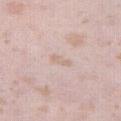Part of a total-body skin-imaging series; this lesion was reviewed on a skin check and was not flagged for biopsy. This image is a 15 mm lesion crop taken from a total-body photograph. The lesion is on the right thigh. A female patient aged 23–27. The tile uses white-light illumination. Longest diameter approximately 3 mm.